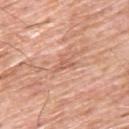Captured during whole-body skin photography for melanoma surveillance; the lesion was not biopsied. A region of skin cropped from a whole-body photographic capture, roughly 15 mm wide. About 2.5 mm across. The subject is a male approximately 60 years of age. The lesion is on the upper back. Captured under white-light illumination.